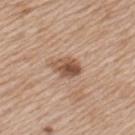Assessment: Imaged during a routine full-body skin examination; the lesion was not biopsied and no histopathology is available. Background: Automated image analysis of the tile measured an area of roughly 6.5 mm², an eccentricity of roughly 0.75, and a symmetry-axis asymmetry near 0.2. Cropped from a total-body skin-imaging series; the visible field is about 15 mm. On the left upper arm. The recorded lesion diameter is about 4 mm. The tile uses white-light illumination. A female subject, approximately 40 years of age.